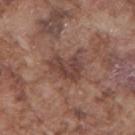biopsy_status: not biopsied; imaged during a skin examination
site: right upper arm
lesion_size:
  long_diameter_mm_approx: 4.0
automated_metrics:
  eccentricity: 0.75
  shape_asymmetry: 0.4
  vs_skin_contrast_norm: 7.5
  border_irregularity_0_10: 4.0
  color_variation_0_10: 3.5
  peripheral_color_asymmetry: 1.5
lighting: white-light
patient:
  sex: male
  age_approx: 75
image:
  source: total-body photography crop
  field_of_view_mm: 15